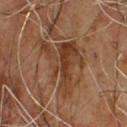Case summary:
- follow-up · total-body-photography surveillance lesion; no biopsy
- size · ≈7.5 mm
- automated lesion analysis · an area of roughly 22 mm² and a symmetry-axis asymmetry near 0.65; a border-irregularity rating of about 9.5/10 and radial color variation of about 2; an automated nevus-likeness rating near 0 out of 100 and a lesion-detection confidence of about 70/100
- illumination · cross-polarized illumination
- image · ~15 mm tile from a whole-body skin photo
- patient · male, roughly 65 years of age